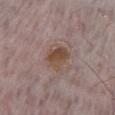patient: male, about 75 years old | illumination: white-light | image source: 15 mm crop, total-body photography | body site: the leg.